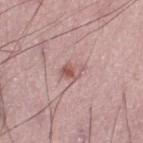Assessment: Part of a total-body skin-imaging series; this lesion was reviewed on a skin check and was not flagged for biopsy. Clinical summary: A 15 mm close-up tile from a total-body photography series done for melanoma screening. On the leg. Automated tile analysis of the lesion measured a lesion area of about 4 mm², a shape eccentricity near 0.75, and a shape-asymmetry score of about 0.25 (0 = symmetric). The software also gave a border-irregularity rating of about 2.5/10, a color-variation rating of about 6/10, and peripheral color asymmetry of about 2. The analysis additionally found a classifier nevus-likeness of about 35/100 and a detector confidence of about 100 out of 100 that the crop contains a lesion. The tile uses white-light illumination. A male patient approximately 60 years of age. The recorded lesion diameter is about 2.5 mm.